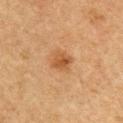Clinical impression:
Captured during whole-body skin photography for melanoma surveillance; the lesion was not biopsied.
Context:
Cropped from a whole-body photographic skin survey; the tile spans about 15 mm. A male patient approximately 75 years of age. The total-body-photography lesion software estimated a border-irregularity index near 2/10, a color-variation rating of about 2.5/10, and a peripheral color-asymmetry measure near 1. Located on the chest. Captured under cross-polarized illumination.